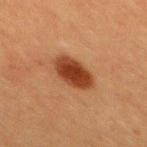follow-up — catalogued during a skin exam; not biopsied | subject — male, about 40 years old | size — about 4 mm | acquisition — 15 mm crop, total-body photography | tile lighting — cross-polarized | body site — the mid back | TBP lesion metrics — a lesion area of about 11 mm² and two-axis asymmetry of about 0.15; a normalized lesion–skin contrast near 11.5; a border-irregularity index near 1.5/10, a within-lesion color-variation index near 3.5/10, and peripheral color asymmetry of about 1; a nevus-likeness score of about 100/100 and a lesion-detection confidence of about 100/100.